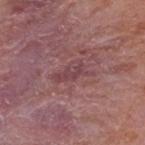Assessment:
This lesion was catalogued during total-body skin photography and was not selected for biopsy.
Background:
A lesion tile, about 15 mm wide, cut from a 3D total-body photograph. The tile uses white-light illumination. Measured at roughly 4.5 mm in maximum diameter. A male patient, approximately 65 years of age. On the mid back.